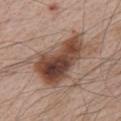Recorded during total-body skin imaging; not selected for excision or biopsy. Located on the front of the torso. The subject is a male in their mid- to late 60s. Approximately 9 mm at its widest. Cropped from a whole-body photographic skin survey; the tile spans about 15 mm.